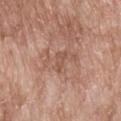Captured during whole-body skin photography for melanoma surveillance; the lesion was not biopsied. A region of skin cropped from a whole-body photographic capture, roughly 15 mm wide. The tile uses white-light illumination. The lesion is located on the upper back. A male subject aged 63–67. About 2.5 mm across. An algorithmic analysis of the crop reported a mean CIELAB color near L≈53 a*≈21 b*≈29, roughly 7 lightness units darker than nearby skin, and a normalized lesion–skin contrast near 5. It also reported a nevus-likeness score of about 0/100 and a lesion-detection confidence of about 100/100.